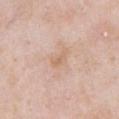| key | value |
|---|---|
| workup | no biopsy performed (imaged during a skin exam) |
| site | the abdomen |
| subject | male, aged 53 to 57 |
| image | ~15 mm tile from a whole-body skin photo |
| tile lighting | white-light illumination |
| lesion diameter | ~2.5 mm (longest diameter) |
| TBP lesion metrics | a footprint of about 3 mm² and an eccentricity of roughly 0.85; a lesion–skin lightness drop of about 6; a nevus-likeness score of about 0/100 and lesion-presence confidence of about 100/100 |